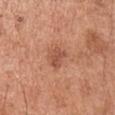Impression:
No biopsy was performed on this lesion — it was imaged during a full skin examination and was not determined to be concerning.
Background:
From the right upper arm. A male patient, aged around 65. This image is a 15 mm lesion crop taken from a total-body photograph.